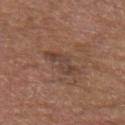| key | value |
|---|---|
| biopsy status | no biopsy performed (imaged during a skin exam) |
| patient | male, aged 78–82 |
| image source | 15 mm crop, total-body photography |
| anatomic site | the upper back |